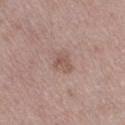Case summary:
– notes: catalogued during a skin exam; not biopsied
– imaging modality: 15 mm crop, total-body photography
– anatomic site: the right thigh
– subject: female, approximately 50 years of age